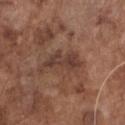The lesion was tiled from a total-body skin photograph and was not biopsied.
The lesion is on the chest.
The subject is a male aged around 75.
A lesion tile, about 15 mm wide, cut from a 3D total-body photograph.
Captured under white-light illumination.